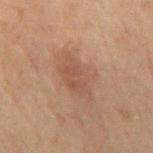Q: Was this lesion biopsied?
A: catalogued during a skin exam; not biopsied
Q: Lesion location?
A: the mid back
Q: How was this image acquired?
A: 15 mm crop, total-body photography
Q: What are the patient's age and sex?
A: male, approximately 70 years of age